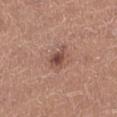Case summary:
* biopsy status: no biopsy performed (imaged during a skin exam)
* acquisition: ~15 mm crop, total-body skin-cancer survey
* site: the left lower leg
* size: ≈3 mm
* subject: female, in their 40s